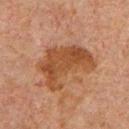Notes:
• patient — male, aged around 60
• anatomic site — the chest
• lesion diameter — ~6.5 mm (longest diameter)
• imaging modality — 15 mm crop, total-body photography
• illumination — cross-polarized illumination